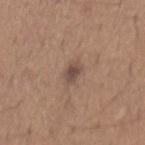Q: What are the patient's age and sex?
A: female, aged 23–27
Q: How was the tile lit?
A: white-light
Q: Lesion location?
A: the back
Q: What kind of image is this?
A: ~15 mm tile from a whole-body skin photo
Q: What is the lesion's diameter?
A: about 3 mm
Q: Automated lesion metrics?
A: a border-irregularity index near 2.5/10, internal color variation of about 3 on a 0–10 scale, and peripheral color asymmetry of about 1